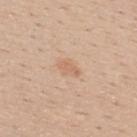Clinical impression:
The lesion was tiled from a total-body skin photograph and was not biopsied.
Acquisition and patient details:
Cropped from a total-body skin-imaging series; the visible field is about 15 mm. The tile uses white-light illumination. About 2.5 mm across. The lesion is located on the upper back. A male patient, aged approximately 30.